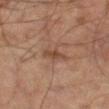Case summary:
- follow-up — imaged on a skin check; not biopsied
- location — the leg
- imaging modality — total-body-photography crop, ~15 mm field of view
- lesion size — ~3 mm (longest diameter)
- patient — male, aged approximately 65